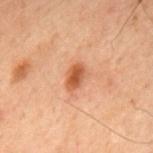No biopsy was performed on this lesion — it was imaged during a full skin examination and was not determined to be concerning. Longest diameter approximately 3 mm. A lesion tile, about 15 mm wide, cut from a 3D total-body photograph. The lesion is on the back. A male patient aged approximately 60. This is a cross-polarized tile.